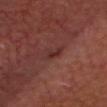{
  "biopsy_status": "not biopsied; imaged during a skin examination",
  "automated_metrics": {
    "eccentricity": 0.85,
    "shape_asymmetry": 0.4,
    "cielab_L": 29,
    "cielab_a": 21,
    "cielab_b": 20,
    "vs_skin_darker_L": 6.0,
    "vs_skin_contrast_norm": 6.5,
    "border_irregularity_0_10": 4.5,
    "peripheral_color_asymmetry": 0.5
  },
  "lighting": "cross-polarized",
  "patient": {
    "sex": "male",
    "age_approx": 65
  },
  "lesion_size": {
    "long_diameter_mm_approx": 3.0
  },
  "site": "head or neck",
  "image": {
    "source": "total-body photography crop",
    "field_of_view_mm": 15
  }
}